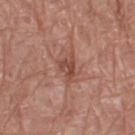  biopsy_status: not biopsied; imaged during a skin examination
  site: right thigh
  patient:
    sex: male
    age_approx: 70
  lesion_size:
    long_diameter_mm_approx: 3.5
  image:
    source: total-body photography crop
    field_of_view_mm: 15
  lighting: white-light